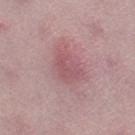Notes:
* workup: imaged on a skin check; not biopsied
* tile lighting: white-light illumination
* acquisition: ~15 mm crop, total-body skin-cancer survey
* diameter: ~4 mm (longest diameter)
* patient: female, in their 50s
* TBP lesion metrics: an outline eccentricity of about 0.8 (0 = round, 1 = elongated) and two-axis asymmetry of about 0.25; a classifier nevus-likeness of about 0/100
* body site: the right thigh